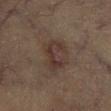Recorded during total-body skin imaging; not selected for excision or biopsy.
Automated image analysis of the tile measured an area of roughly 13 mm², an outline eccentricity of about 0.7 (0 = round, 1 = elongated), and two-axis asymmetry of about 0.25. The software also gave an average lesion color of about L≈30 a*≈12 b*≈19 (CIELAB), roughly 6 lightness units darker than nearby skin, and a normalized lesion–skin contrast near 6.5. The software also gave a border-irregularity index near 3/10 and a color-variation rating of about 4.5/10. And it measured an automated nevus-likeness rating near 5 out of 100 and a lesion-detection confidence of about 100/100.
Imaged with cross-polarized lighting.
A 15 mm close-up tile from a total-body photography series done for melanoma screening.
About 5 mm across.
The subject is a male about 60 years old.
The lesion is on the left lower leg.